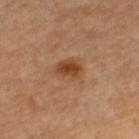Recorded during total-body skin imaging; not selected for excision or biopsy.
A male patient, roughly 65 years of age.
The lesion is located on the right lower leg.
A 15 mm close-up tile from a total-body photography series done for melanoma screening.
Automated tile analysis of the lesion measured a lesion-to-skin contrast of about 8.5 (normalized; higher = more distinct). It also reported an automated nevus-likeness rating near 95 out of 100 and lesion-presence confidence of about 100/100.
About 3 mm across.
This is a cross-polarized tile.